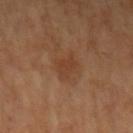Acquisition and patient details:
The lesion's longest dimension is about 3 mm. The subject is a female aged around 65. Imaged with cross-polarized lighting. Cropped from a whole-body photographic skin survey; the tile spans about 15 mm. The lesion is on the arm.